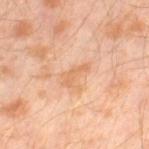The lesion was tiled from a total-body skin photograph and was not biopsied. About 3 mm across. A close-up tile cropped from a whole-body skin photograph, about 15 mm across. Located on the left thigh. This is a cross-polarized tile. A male subject aged 28 to 32.